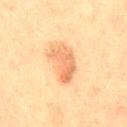The lesion was tiled from a total-body skin photograph and was not biopsied. The lesion-visualizer software estimated a footprint of about 10 mm², a shape eccentricity near 0.85, and two-axis asymmetry of about 0.4. And it measured a border-irregularity rating of about 4.5/10, internal color variation of about 4.5 on a 0–10 scale, and a peripheral color-asymmetry measure near 1.5. A lesion tile, about 15 mm wide, cut from a 3D total-body photograph. The patient is a female in their mid- to late 50s. This is a cross-polarized tile. Approximately 5 mm at its widest. The lesion is located on the right thigh.